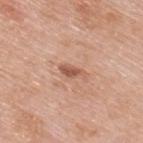Imaged during a routine full-body skin examination; the lesion was not biopsied and no histopathology is available.
A 15 mm close-up extracted from a 3D total-body photography capture.
A male patient, aged 78–82.
Located on the back.